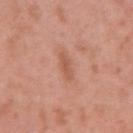Clinical summary:
The lesion-visualizer software estimated a within-lesion color-variation index near 2/10 and radial color variation of about 0.5. A close-up tile cropped from a whole-body skin photograph, about 15 mm across. Located on the right upper arm. The recorded lesion diameter is about 3 mm. A female subject, approximately 40 years of age.